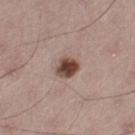notes: no biopsy performed (imaged during a skin exam)
acquisition: total-body-photography crop, ~15 mm field of view
illumination: white-light illumination
patient: male, roughly 65 years of age
lesion diameter: ≈3 mm
location: the left thigh
TBP lesion metrics: an area of roughly 5.5 mm², a shape eccentricity near 0.45, and a symmetry-axis asymmetry near 0.15; a lesion color around L≈45 a*≈20 b*≈23 in CIELAB, roughly 17 lightness units darker than nearby skin, and a normalized border contrast of about 12.5